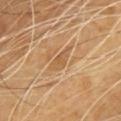Clinical impression:
Imaged during a routine full-body skin examination; the lesion was not biopsied and no histopathology is available.
Acquisition and patient details:
A male subject aged around 60. A close-up tile cropped from a whole-body skin photograph, about 15 mm across. Located on the chest.